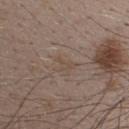Part of a total-body skin-imaging series; this lesion was reviewed on a skin check and was not flagged for biopsy. A roughly 15 mm field-of-view crop from a total-body skin photograph. On the upper back. The tile uses white-light illumination. The patient is a male aged around 50.